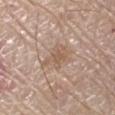| field | value |
|---|---|
| notes | imaged on a skin check; not biopsied |